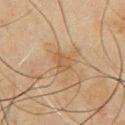Impression: Part of a total-body skin-imaging series; this lesion was reviewed on a skin check and was not flagged for biopsy. Background: Cropped from a total-body skin-imaging series; the visible field is about 15 mm. The lesion is on the chest. A male subject aged 43 to 47.